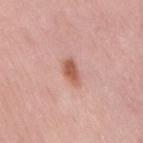<case>
<biopsy_status>not biopsied; imaged during a skin examination</biopsy_status>
<site>right thigh</site>
<lesion_size>
  <long_diameter_mm_approx>3.5</long_diameter_mm_approx>
</lesion_size>
<patient>
  <sex>female</sex>
  <age_approx>50</age_approx>
</patient>
<automated_metrics>
  <cielab_L>58</cielab_L>
  <cielab_a>25</cielab_a>
  <cielab_b>30</cielab_b>
  <vs_skin_darker_L>12.0</vs_skin_darker_L>
  <vs_skin_contrast_norm>8.5</vs_skin_contrast_norm>
  <border_irregularity_0_10>2.5</border_irregularity_0_10>
  <color_variation_0_10>2.5</color_variation_0_10>
  <peripheral_color_asymmetry>1.0</peripheral_color_asymmetry>
</automated_metrics>
<lighting>white-light</lighting>
<image>
  <source>total-body photography crop</source>
  <field_of_view_mm>15</field_of_view_mm>
</image>
</case>